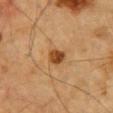biopsy_status: not biopsied; imaged during a skin examination
image:
  source: total-body photography crop
  field_of_view_mm: 15
patient:
  sex: male
  age_approx: 85+
site: chest